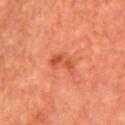Q: Is there a histopathology result?
A: catalogued during a skin exam; not biopsied
Q: How large is the lesion?
A: ~3.5 mm (longest diameter)
Q: What is the imaging modality?
A: ~15 mm tile from a whole-body skin photo
Q: Lesion location?
A: the chest
Q: What lighting was used for the tile?
A: cross-polarized
Q: What are the patient's age and sex?
A: male, aged approximately 65